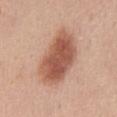workup: imaged on a skin check; not biopsied
image source: total-body-photography crop, ~15 mm field of view
lighting: white-light illumination
location: the back
subject: female, aged 43 to 47
lesion size: ≈8 mm